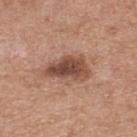Case summary:
- biopsy status — total-body-photography surveillance lesion; no biopsy
- size — ~5.5 mm (longest diameter)
- tile lighting — white-light illumination
- body site — the upper back
- patient — male, aged 78–82
- image source — 15 mm crop, total-body photography